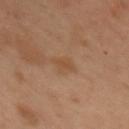The lesion was tiled from a total-body skin photograph and was not biopsied.
A lesion tile, about 15 mm wide, cut from a 3D total-body photograph.
The tile uses cross-polarized illumination.
Longest diameter approximately 2.5 mm.
The lesion is located on the upper back.
The patient is a female about 55 years old.
Automated tile analysis of the lesion measured an area of roughly 3 mm², an eccentricity of roughly 0.85, and two-axis asymmetry of about 0.2. It also reported border irregularity of about 2 on a 0–10 scale, internal color variation of about 0.5 on a 0–10 scale, and a peripheral color-asymmetry measure near 0.5. The software also gave an automated nevus-likeness rating near 0 out of 100.